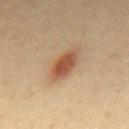Assessment: Captured during whole-body skin photography for melanoma surveillance; the lesion was not biopsied. Clinical summary: The lesion is on the mid back. A male subject, aged approximately 40. A lesion tile, about 15 mm wide, cut from a 3D total-body photograph.